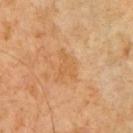The lesion was tiled from a total-body skin photograph and was not biopsied.
Cropped from a total-body skin-imaging series; the visible field is about 15 mm.
A male patient, approximately 65 years of age.
Measured at roughly 3.5 mm in maximum diameter.